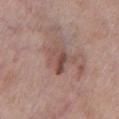Q: What kind of image is this?
A: ~15 mm tile from a whole-body skin photo
Q: Illumination type?
A: white-light
Q: What did automated image analysis measure?
A: a lesion area of about 8.5 mm² and a shape-asymmetry score of about 0.35 (0 = symmetric); a mean CIELAB color near L≈49 a*≈19 b*≈23, a lesion–skin lightness drop of about 9, and a normalized border contrast of about 6.5; border irregularity of about 4.5 on a 0–10 scale, a within-lesion color-variation index near 7/10, and a peripheral color-asymmetry measure near 2.5
Q: Who is the patient?
A: male, aged 58–62
Q: Where on the body is the lesion?
A: the chest